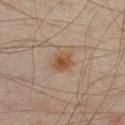<lesion>
<site>right lower leg</site>
<automated_metrics>
  <area_mm2_approx>5.0</area_mm2_approx>
  <eccentricity>0.6</eccentricity>
  <shape_asymmetry>0.25</shape_asymmetry>
  <cielab_L>42</cielab_L>
  <cielab_a>16</cielab_a>
  <cielab_b>29</cielab_b>
  <vs_skin_darker_L>8.0</vs_skin_darker_L>
  <vs_skin_contrast_norm>8.5</vs_skin_contrast_norm>
  <border_irregularity_0_10>2.5</border_irregularity_0_10>
  <color_variation_0_10>3.5</color_variation_0_10>
  <peripheral_color_asymmetry>1.0</peripheral_color_asymmetry>
</automated_metrics>
<patient>
  <sex>male</sex>
  <age_approx>45</age_approx>
</patient>
<lighting>cross-polarized</lighting>
<lesion_size>
  <long_diameter_mm_approx>2.5</long_diameter_mm_approx>
</lesion_size>
<image>
  <source>total-body photography crop</source>
  <field_of_view_mm>15</field_of_view_mm>
</image>
</lesion>